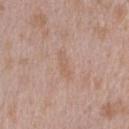Q: Was this lesion biopsied?
A: catalogued during a skin exam; not biopsied
Q: What kind of image is this?
A: total-body-photography crop, ~15 mm field of view
Q: Lesion location?
A: the arm
Q: What are the patient's age and sex?
A: male, roughly 45 years of age
Q: Illumination type?
A: white-light
Q: How large is the lesion?
A: about 3.5 mm